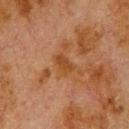<tbp_lesion>
  <biopsy_status>not biopsied; imaged during a skin examination</biopsy_status>
  <image>
    <source>total-body photography crop</source>
    <field_of_view_mm>15</field_of_view_mm>
  </image>
  <lighting>cross-polarized</lighting>
  <patient>
    <sex>male</sex>
    <age_approx>80</age_approx>
  </patient>
  <site>upper back</site>
  <lesion_size>
    <long_diameter_mm_approx>2.5</long_diameter_mm_approx>
  </lesion_size>
</tbp_lesion>